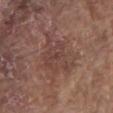Q: Was a biopsy performed?
A: total-body-photography surveillance lesion; no biopsy
Q: Who is the patient?
A: male, roughly 80 years of age
Q: How was this image acquired?
A: 15 mm crop, total-body photography
Q: What is the anatomic site?
A: the mid back